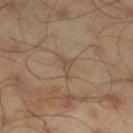biopsy status: imaged on a skin check; not biopsied
patient: male, aged 43 to 47
acquisition: total-body-photography crop, ~15 mm field of view
illumination: cross-polarized
anatomic site: the leg
lesion diameter: ~3 mm (longest diameter)